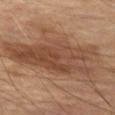  biopsy_status: not biopsied; imaged during a skin examination
  patient:
    sex: male
    age_approx: 70
  lesion_size:
    long_diameter_mm_approx: 13.0
  image:
    source: total-body photography crop
    field_of_view_mm: 15
  site: left thigh
  automated_metrics:
    border_irregularity_0_10: 6.5
    color_variation_0_10: 5.5
    peripheral_color_asymmetry: 2.0
    nevus_likeness_0_100: 45
  lighting: cross-polarized